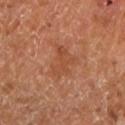Impression:
The lesion was photographed on a routine skin check and not biopsied; there is no pathology result.
Background:
Cropped from a total-body skin-imaging series; the visible field is about 15 mm. The tile uses cross-polarized illumination. The lesion-visualizer software estimated an area of roughly 8.5 mm² and a shape-asymmetry score of about 0.4 (0 = symmetric). It also reported a mean CIELAB color near L≈47 a*≈25 b*≈34, roughly 6 lightness units darker than nearby skin, and a normalized lesion–skin contrast near 5. It also reported a nevus-likeness score of about 0/100. The patient is roughly 65 years of age. Longest diameter approximately 3.5 mm. The lesion is on the left lower leg.